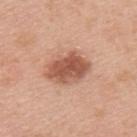Captured during whole-body skin photography for melanoma surveillance; the lesion was not biopsied.
The lesion is located on the upper back.
The tile uses white-light illumination.
Cropped from a total-body skin-imaging series; the visible field is about 15 mm.
A male patient, about 35 years old.
The recorded lesion diameter is about 5.5 mm.